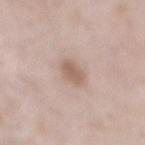biopsy_status: not biopsied; imaged during a skin examination
patient:
  sex: male
  age_approx: 55
lighting: white-light
site: mid back
lesion_size:
  long_diameter_mm_approx: 2.5
image:
  source: total-body photography crop
  field_of_view_mm: 15